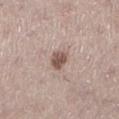Acquisition and patient details:
A roughly 15 mm field-of-view crop from a total-body skin photograph. Longest diameter approximately 2.5 mm. A female patient, aged approximately 40. The tile uses white-light illumination. On the left lower leg. An algorithmic analysis of the crop reported an area of roughly 4.5 mm² and a symmetry-axis asymmetry near 0.25. The analysis additionally found a nevus-likeness score of about 75/100 and a lesion-detection confidence of about 100/100.